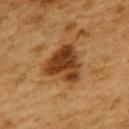| key | value |
|---|---|
| notes | catalogued during a skin exam; not biopsied |
| location | the upper back |
| TBP lesion metrics | an average lesion color of about L≈41 a*≈23 b*≈39 (CIELAB), about 15 CIELAB-L* units darker than the surrounding skin, and a normalized lesion–skin contrast near 11.5; a nevus-likeness score of about 90/100 |
| acquisition | 15 mm crop, total-body photography |
| patient | female, roughly 55 years of age |
| diameter | about 4.5 mm |